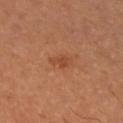An algorithmic analysis of the crop reported a lesion area of about 3.5 mm², an eccentricity of roughly 0.8, and a shape-asymmetry score of about 0.3 (0 = symmetric). The analysis additionally found a mean CIELAB color near L≈47 a*≈26 b*≈36 and a normalized border contrast of about 5.5. And it measured a border-irregularity index near 3/10 and radial color variation of about 0.5. It also reported a classifier nevus-likeness of about 5/100 and lesion-presence confidence of about 100/100. Approximately 2.5 mm at its widest. A 15 mm close-up tile from a total-body photography series done for melanoma screening. Located on the arm. A male patient approximately 65 years of age. This is a cross-polarized tile.